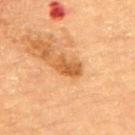notes — imaged on a skin check; not biopsied
body site — the upper back
lighting — cross-polarized
patient — female, approximately 70 years of age
imaging modality — 15 mm crop, total-body photography
lesion size — ≈3.5 mm